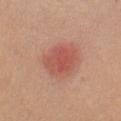Notes:
* workup · catalogued during a skin exam; not biopsied
* site · the left upper arm
* subject · female, approximately 50 years of age
* image source · ~15 mm crop, total-body skin-cancer survey